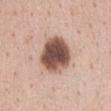A 15 mm close-up tile from a total-body photography series done for melanoma screening.
The patient is a female in their mid- to late 40s.
The lesion is on the chest.
Measured at roughly 5.5 mm in maximum diameter.